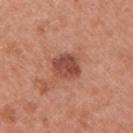Captured during whole-body skin photography for melanoma surveillance; the lesion was not biopsied. A close-up tile cropped from a whole-body skin photograph, about 15 mm across. The lesion is located on the right upper arm. Imaged with white-light lighting. Longest diameter approximately 3.5 mm. The patient is a female about 40 years old.